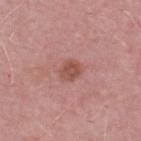Part of a total-body skin-imaging series; this lesion was reviewed on a skin check and was not flagged for biopsy.
The lesion is on the back.
Measured at roughly 2.5 mm in maximum diameter.
Captured under white-light illumination.
An algorithmic analysis of the crop reported a color-variation rating of about 2/10 and peripheral color asymmetry of about 1. And it measured an automated nevus-likeness rating near 30 out of 100 and lesion-presence confidence of about 100/100.
A male subject, aged 48–52.
A roughly 15 mm field-of-view crop from a total-body skin photograph.